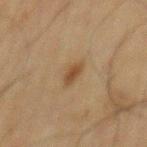Impression: The lesion was tiled from a total-body skin photograph and was not biopsied. Acquisition and patient details: The subject is a male about 70 years old. The lesion is located on the mid back. About 3 mm across. A 15 mm close-up extracted from a 3D total-body photography capture.